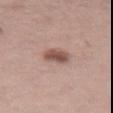This lesion was catalogued during total-body skin photography and was not selected for biopsy.
Automated image analysis of the tile measured a lesion color around L≈52 a*≈20 b*≈24 in CIELAB, a lesion–skin lightness drop of about 13, and a normalized lesion–skin contrast near 9.
The lesion is on the left thigh.
A 15 mm close-up extracted from a 3D total-body photography capture.
A female subject, in their mid- to late 40s.